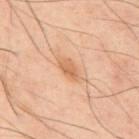biopsy status = imaged on a skin check; not biopsied.